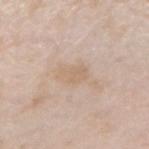notes: no biopsy performed (imaged during a skin exam) | TBP lesion metrics: a lesion area of about 4.5 mm², an eccentricity of roughly 0.8, and two-axis asymmetry of about 0.3; roughly 6 lightness units darker than nearby skin and a normalized lesion–skin contrast near 4.5; a border-irregularity index near 3/10, a color-variation rating of about 1/10, and peripheral color asymmetry of about 0.5 | location: the right forearm | subject: male, about 45 years old | imaging modality: ~15 mm crop, total-body skin-cancer survey | illumination: white-light illumination | size: ~3 mm (longest diameter).